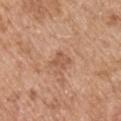| field | value |
|---|---|
| subject | male, approximately 55 years of age |
| lesion size | about 2.5 mm |
| location | the left upper arm |
| TBP lesion metrics | a lesion area of about 3.5 mm², a shape eccentricity near 0.6, and a symmetry-axis asymmetry near 0.4; a lesion color around L≈55 a*≈22 b*≈32 in CIELAB and about 8 CIELAB-L* units darker than the surrounding skin; border irregularity of about 4 on a 0–10 scale, a color-variation rating of about 1.5/10, and radial color variation of about 0.5 |
| image source | ~15 mm crop, total-body skin-cancer survey |
| illumination | white-light illumination |